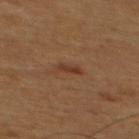Impression: This lesion was catalogued during total-body skin photography and was not selected for biopsy. Image and clinical context: A lesion tile, about 15 mm wide, cut from a 3D total-body photograph. This is a cross-polarized tile. The lesion is on the mid back. A male patient, in their 60s. Longest diameter approximately 2.5 mm.